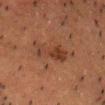{"biopsy_status": "not biopsied; imaged during a skin examination", "lighting": "cross-polarized", "lesion_size": {"long_diameter_mm_approx": 6.0}, "site": "head or neck", "automated_metrics": {"border_irregularity_0_10": 5.5, "color_variation_0_10": 4.5, "peripheral_color_asymmetry": 1.5, "nevus_likeness_0_100": 40, "lesion_detection_confidence_0_100": 100}, "patient": {"sex": "male", "age_approx": 50}, "image": {"source": "total-body photography crop", "field_of_view_mm": 15}}